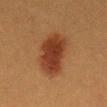Case summary:
• workup: catalogued during a skin exam; not biopsied
• site: the mid back
• diameter: ~6.5 mm (longest diameter)
• acquisition: total-body-photography crop, ~15 mm field of view
• illumination: cross-polarized illumination
• patient: female, about 40 years old